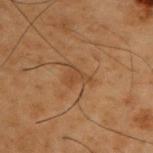Impression:
No biopsy was performed on this lesion — it was imaged during a full skin examination and was not determined to be concerning.
Acquisition and patient details:
The total-body-photography lesion software estimated a mean CIELAB color near L≈37 a*≈17 b*≈30 and a normalized lesion–skin contrast near 5. The analysis additionally found border irregularity of about 5.5 on a 0–10 scale, internal color variation of about 1 on a 0–10 scale, and radial color variation of about 0.5. Located on the upper back. A 15 mm close-up tile from a total-body photography series done for melanoma screening. A male patient, aged 53–57. Imaged with cross-polarized lighting. Approximately 3 mm at its widest.